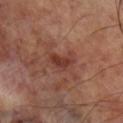illumination = cross-polarized illumination | acquisition = 15 mm crop, total-body photography | patient = male, about 70 years old | diameter = ≈3 mm | site = the right lower leg.